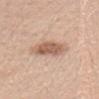Captured during whole-body skin photography for melanoma surveillance; the lesion was not biopsied.
Cropped from a total-body skin-imaging series; the visible field is about 15 mm.
A female patient aged around 25.
Captured under white-light illumination.
Located on the head or neck.
Longest diameter approximately 4.5 mm.
Automated image analysis of the tile measured a lesion area of about 9.5 mm², an outline eccentricity of about 0.85 (0 = round, 1 = elongated), and two-axis asymmetry of about 0.15. The analysis additionally found a mean CIELAB color near L≈60 a*≈20 b*≈30, roughly 13 lightness units darker than nearby skin, and a normalized border contrast of about 8. The software also gave a nevus-likeness score of about 75/100 and a lesion-detection confidence of about 100/100.